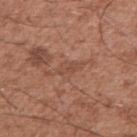image source: ~15 mm crop, total-body skin-cancer survey
anatomic site: the right upper arm
patient: male, approximately 50 years of age
lesion size: about 2.5 mm
lighting: white-light